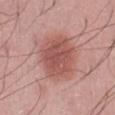Captured during whole-body skin photography for melanoma surveillance; the lesion was not biopsied.
From the abdomen.
A male subject, approximately 50 years of age.
Cropped from a total-body skin-imaging series; the visible field is about 15 mm.
The total-body-photography lesion software estimated a lesion–skin lightness drop of about 11. The software also gave an automated nevus-likeness rating near 100 out of 100.
Longest diameter approximately 6 mm.
The tile uses white-light illumination.